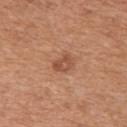Part of a total-body skin-imaging series; this lesion was reviewed on a skin check and was not flagged for biopsy. A roughly 15 mm field-of-view crop from a total-body skin photograph. The lesion is located on the upper back. Automated tile analysis of the lesion measured a lesion area of about 4 mm², an outline eccentricity of about 0.75 (0 = round, 1 = elongated), and a symmetry-axis asymmetry near 0.2. The tile uses white-light illumination. The patient is a female in their 50s. Measured at roughly 2.5 mm in maximum diameter.